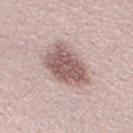Acquisition and patient details: An algorithmic analysis of the crop reported a shape eccentricity near 0.7 and a symmetry-axis asymmetry near 0.2. And it measured a border-irregularity rating of about 2.5/10, a color-variation rating of about 3.5/10, and radial color variation of about 1. About 5.5 mm across. Cropped from a total-body skin-imaging series; the visible field is about 15 mm. The tile uses white-light illumination. A male subject in their 30s.